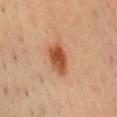biopsy status = catalogued during a skin exam; not biopsied | patient = male, aged 38 to 42 | acquisition = 15 mm crop, total-body photography | lighting = cross-polarized illumination | body site = the front of the torso.